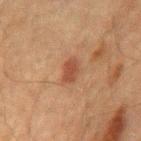follow-up: no biopsy performed (imaged during a skin exam).